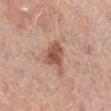Clinical impression: No biopsy was performed on this lesion — it was imaged during a full skin examination and was not determined to be concerning. Clinical summary: About 4 mm across. The subject is a female in their mid-60s. The lesion is located on the right lower leg. Imaged with white-light lighting. A roughly 15 mm field-of-view crop from a total-body skin photograph.